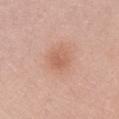location: the chest | image source: 15 mm crop, total-body photography | subject: female, aged approximately 50.